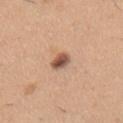A male subject, about 40 years old.
The tile uses white-light illumination.
The total-body-photography lesion software estimated a border-irregularity index near 1.5/10, a within-lesion color-variation index near 5/10, and peripheral color asymmetry of about 1.5. The analysis additionally found an automated nevus-likeness rating near 95 out of 100 and a lesion-detection confidence of about 100/100.
A roughly 15 mm field-of-view crop from a total-body skin photograph.
About 2.5 mm across.
On the left upper arm.